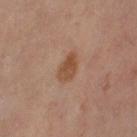Q: Was this lesion biopsied?
A: catalogued during a skin exam; not biopsied
Q: Automated lesion metrics?
A: a shape eccentricity near 0.85 and a symmetry-axis asymmetry near 0.2; an average lesion color of about L≈47 a*≈20 b*≈31 (CIELAB) and a lesion-to-skin contrast of about 8 (normalized; higher = more distinct); a detector confidence of about 100 out of 100 that the crop contains a lesion
Q: How was this image acquired?
A: ~15 mm crop, total-body skin-cancer survey
Q: How large is the lesion?
A: ~4 mm (longest diameter)
Q: Lesion location?
A: the right thigh
Q: What lighting was used for the tile?
A: cross-polarized
Q: Patient demographics?
A: female, aged 48 to 52